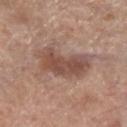Q: Is there a histopathology result?
A: total-body-photography surveillance lesion; no biopsy
Q: Who is the patient?
A: male, aged 68–72
Q: What kind of image is this?
A: total-body-photography crop, ~15 mm field of view
Q: Automated lesion metrics?
A: a lesion area of about 18 mm² and two-axis asymmetry of about 0.35; about 11 CIELAB-L* units darker than the surrounding skin and a lesion-to-skin contrast of about 7.5 (normalized; higher = more distinct)
Q: Illumination type?
A: white-light illumination
Q: What is the anatomic site?
A: the right lower leg